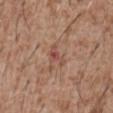This lesion was catalogued during total-body skin photography and was not selected for biopsy. The lesion's longest dimension is about 3 mm. A roughly 15 mm field-of-view crop from a total-body skin photograph. Imaged with white-light lighting. The lesion is on the abdomen. The patient is a male aged 43–47.